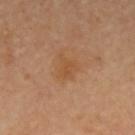This lesion was catalogued during total-body skin photography and was not selected for biopsy. A male patient roughly 65 years of age. This image is a 15 mm lesion crop taken from a total-body photograph. The lesion is located on the left upper arm.